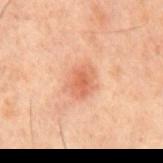Captured during whole-body skin photography for melanoma surveillance; the lesion was not biopsied.
Located on the mid back.
A lesion tile, about 15 mm wide, cut from a 3D total-body photograph.
A male subject, roughly 60 years of age.